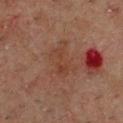Assessment:
Captured during whole-body skin photography for melanoma surveillance; the lesion was not biopsied.
Background:
The lesion is on the chest. A male patient, aged 48 to 52. This image is a 15 mm lesion crop taken from a total-body photograph. The recorded lesion diameter is about 4 mm.